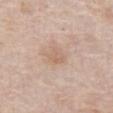Impression: No biopsy was performed on this lesion — it was imaged during a full skin examination and was not determined to be concerning. Clinical summary: The lesion is located on the abdomen. The subject is a male aged 63 to 67. Captured under white-light illumination. Cropped from a total-body skin-imaging series; the visible field is about 15 mm.